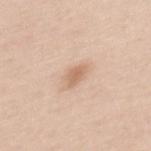Q: What kind of image is this?
A: total-body-photography crop, ~15 mm field of view
Q: Patient demographics?
A: female, in their mid- to late 30s
Q: Where on the body is the lesion?
A: the mid back